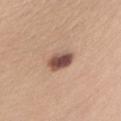Part of a total-body skin-imaging series; this lesion was reviewed on a skin check and was not flagged for biopsy. A female patient, aged around 25. The lesion is on the left upper arm. A lesion tile, about 15 mm wide, cut from a 3D total-body photograph.